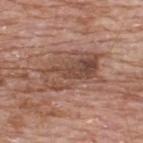Case summary:
• image source: total-body-photography crop, ~15 mm field of view
• size: ~7 mm (longest diameter)
• tile lighting: white-light
• site: the upper back
• patient: male, in their mid- to late 60s